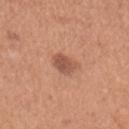Q: Was this lesion biopsied?
A: imaged on a skin check; not biopsied
Q: How was this image acquired?
A: 15 mm crop, total-body photography
Q: Patient demographics?
A: female, aged around 30
Q: What is the anatomic site?
A: the left upper arm
Q: How large is the lesion?
A: about 2.5 mm
Q: What lighting was used for the tile?
A: white-light